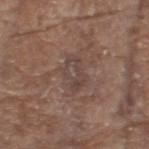The lesion was photographed on a routine skin check and not biopsied; there is no pathology result.
The tile uses white-light illumination.
The patient is a male roughly 80 years of age.
This image is a 15 mm lesion crop taken from a total-body photograph.
On the head or neck.